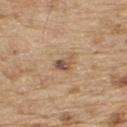Findings:
– follow-up: catalogued during a skin exam; not biopsied
– lesion diameter: ~2.5 mm (longest diameter)
– tile lighting: white-light illumination
– patient: male, about 70 years old
– image: ~15 mm tile from a whole-body skin photo
– body site: the upper back